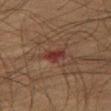notes: no biopsy performed (imaged during a skin exam)
size: ~3.5 mm (longest diameter)
imaging modality: 15 mm crop, total-body photography
patient: male, aged 63 to 67
illumination: cross-polarized illumination
body site: the right thigh